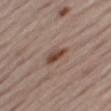Assessment:
No biopsy was performed on this lesion — it was imaged during a full skin examination and was not determined to be concerning.
Background:
The subject is a male roughly 65 years of age. The lesion is located on the right thigh. This image is a 15 mm lesion crop taken from a total-body photograph.